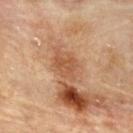{
  "biopsy_status": "not biopsied; imaged during a skin examination",
  "image": {
    "source": "total-body photography crop",
    "field_of_view_mm": 15
  },
  "site": "upper back",
  "automated_metrics": {
    "eccentricity": 0.6,
    "shape_asymmetry": 0.4,
    "border_irregularity_0_10": 4.0,
    "peripheral_color_asymmetry": 1.0
  },
  "patient": {
    "sex": "male",
    "age_approx": "85+"
  },
  "lighting": "cross-polarized"
}